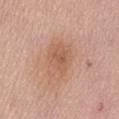A female patient, aged 63–67. Cropped from a whole-body photographic skin survey; the tile spans about 15 mm. The lesion is located on the abdomen. The total-body-photography lesion software estimated an eccentricity of roughly 0.7 and a shape-asymmetry score of about 0.25 (0 = symmetric). The analysis additionally found roughly 8 lightness units darker than nearby skin and a normalized border contrast of about 5.5. Captured under white-light illumination. Approximately 4.5 mm at its widest.